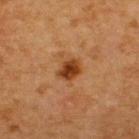Part of a total-body skin-imaging series; this lesion was reviewed on a skin check and was not flagged for biopsy. Located on the upper back. A male subject about 65 years old. This image is a 15 mm lesion crop taken from a total-body photograph.